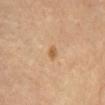Recorded during total-body skin imaging; not selected for excision or biopsy. A female subject approximately 65 years of age. This image is a 15 mm lesion crop taken from a total-body photograph. This is a cross-polarized tile. The recorded lesion diameter is about 2 mm. Located on the front of the torso.